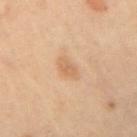Captured during whole-body skin photography for melanoma surveillance; the lesion was not biopsied. The subject is a female approximately 45 years of age. Automated tile analysis of the lesion measured border irregularity of about 2.5 on a 0–10 scale, internal color variation of about 1.5 on a 0–10 scale, and a peripheral color-asymmetry measure near 0.5. It also reported a classifier nevus-likeness of about 20/100 and a detector confidence of about 100 out of 100 that the crop contains a lesion. From the arm. A roughly 15 mm field-of-view crop from a total-body skin photograph.